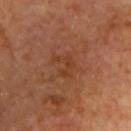The lesion is on the chest. Measured at roughly 3 mm in maximum diameter. A male patient aged 63–67. A close-up tile cropped from a whole-body skin photograph, about 15 mm across.